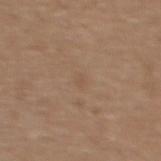notes = imaged on a skin check; not biopsied | anatomic site = the back | tile lighting = white-light illumination | subject = female, aged 43–47 | TBP lesion metrics = an area of roughly 1.5 mm² and a symmetry-axis asymmetry near 0.35; a classifier nevus-likeness of about 0/100 and a lesion-detection confidence of about 100/100 | imaging modality = ~15 mm crop, total-body skin-cancer survey.